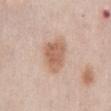The recorded lesion diameter is about 5 mm.
On the abdomen.
The subject is a male aged 73 to 77.
A 15 mm close-up extracted from a 3D total-body photography capture.
This is a white-light tile.